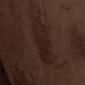Findings:
– biopsy status — imaged on a skin check; not biopsied
– site — the abdomen
– subject — male, aged 68 to 72
– image source — ~15 mm crop, total-body skin-cancer survey
– size — about 7 mm
– tile lighting — white-light